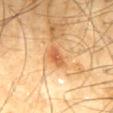| key | value |
|---|---|
| biopsy status | imaged on a skin check; not biopsied |
| size | about 3 mm |
| automated lesion analysis | an area of roughly 4 mm², an eccentricity of roughly 0.8, and a shape-asymmetry score of about 0.3 (0 = symmetric); a nevus-likeness score of about 55/100 and lesion-presence confidence of about 100/100 |
| subject | male, in their mid- to late 60s |
| body site | the mid back |
| acquisition | ~15 mm tile from a whole-body skin photo |
| illumination | cross-polarized illumination |